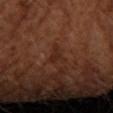Q: Is there a histopathology result?
A: no biopsy performed (imaged during a skin exam)
Q: How large is the lesion?
A: ~2.5 mm (longest diameter)
Q: Lesion location?
A: the right forearm
Q: Who is the patient?
A: female, aged around 65
Q: Illumination type?
A: cross-polarized
Q: What is the imaging modality?
A: 15 mm crop, total-body photography